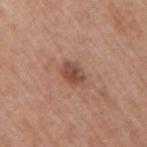Captured during whole-body skin photography for melanoma surveillance; the lesion was not biopsied.
This is a white-light tile.
A region of skin cropped from a whole-body photographic capture, roughly 15 mm wide.
On the right upper arm.
Longest diameter approximately 3 mm.
The subject is a male aged approximately 65.